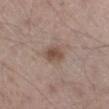This lesion was catalogued during total-body skin photography and was not selected for biopsy.
A male patient, about 55 years old.
Located on the left thigh.
The tile uses white-light illumination.
A region of skin cropped from a whole-body photographic capture, roughly 15 mm wide.
The lesion's longest dimension is about 3 mm.
The total-body-photography lesion software estimated an area of roughly 5 mm² and a shape eccentricity near 0.7. It also reported border irregularity of about 2 on a 0–10 scale, a color-variation rating of about 3/10, and radial color variation of about 1.